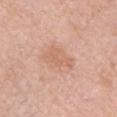Part of a total-body skin-imaging series; this lesion was reviewed on a skin check and was not flagged for biopsy. Captured under white-light illumination. The recorded lesion diameter is about 4.5 mm. Automated tile analysis of the lesion measured an outline eccentricity of about 0.85 (0 = round, 1 = elongated) and a symmetry-axis asymmetry near 0.3. And it measured a lesion color around L≈64 a*≈22 b*≈32 in CIELAB, about 7 CIELAB-L* units darker than the surrounding skin, and a normalized border contrast of about 5. The software also gave border irregularity of about 3.5 on a 0–10 scale, a within-lesion color-variation index near 2.5/10, and radial color variation of about 1. And it measured a nevus-likeness score of about 5/100 and lesion-presence confidence of about 100/100. From the right upper arm. A lesion tile, about 15 mm wide, cut from a 3D total-body photograph. A female subject in their mid- to late 60s.